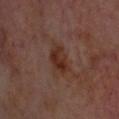{"biopsy_status": "not biopsied; imaged during a skin examination", "image": {"source": "total-body photography crop", "field_of_view_mm": 15}, "patient": {"age_approx": 65}, "site": "head or neck", "lesion_size": {"long_diameter_mm_approx": 4.0}, "lighting": "cross-polarized", "automated_metrics": {"border_irregularity_0_10": 3.0, "color_variation_0_10": 4.5, "nevus_likeness_0_100": 50, "lesion_detection_confidence_0_100": 100}}